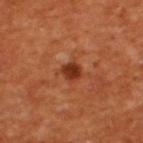<case>
  <image>
    <source>total-body photography crop</source>
    <field_of_view_mm>15</field_of_view_mm>
  </image>
  <site>upper back</site>
  <patient>
    <sex>male</sex>
    <age_approx>55</age_approx>
  </patient>
</case>